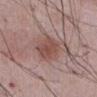Findings:
- notes: no biopsy performed (imaged during a skin exam)
- acquisition: ~15 mm crop, total-body skin-cancer survey
- body site: the abdomen
- image-analysis metrics: a classifier nevus-likeness of about 95/100 and lesion-presence confidence of about 100/100
- lesion size: ~4.5 mm (longest diameter)
- illumination: white-light
- patient: male, aged approximately 55